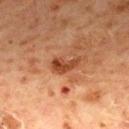Notes:
- notes: catalogued during a skin exam; not biopsied
- subject: male, in their mid-60s
- site: the mid back
- imaging modality: total-body-photography crop, ~15 mm field of view